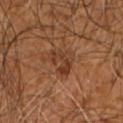<lesion>
<biopsy_status>not biopsied; imaged during a skin examination</biopsy_status>
<patient>
  <sex>male</sex>
  <age_approx>60</age_approx>
</patient>
<lighting>cross-polarized</lighting>
<lesion_size>
  <long_diameter_mm_approx>3.5</long_diameter_mm_approx>
</lesion_size>
<image>
  <source>total-body photography crop</source>
  <field_of_view_mm>15</field_of_view_mm>
</image>
<site>left arm</site>
</lesion>